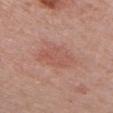This lesion was catalogued during total-body skin photography and was not selected for biopsy.